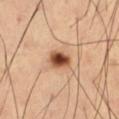{"biopsy_status": "not biopsied; imaged during a skin examination", "patient": {"sex": "male", "age_approx": 65}, "site": "left thigh", "lesion_size": {"long_diameter_mm_approx": 3.0}, "image": {"source": "total-body photography crop", "field_of_view_mm": 15}}